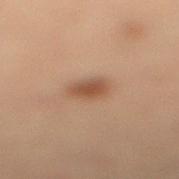Part of a total-body skin-imaging series; this lesion was reviewed on a skin check and was not flagged for biopsy. Imaged with cross-polarized lighting. The lesion's longest dimension is about 2.5 mm. The subject is a male roughly 55 years of age. A 15 mm close-up tile from a total-body photography series done for melanoma screening. The lesion is located on the right lower leg.